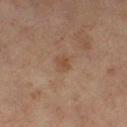Captured during whole-body skin photography for melanoma surveillance; the lesion was not biopsied.
From the left thigh.
This image is a 15 mm lesion crop taken from a total-body photograph.
Approximately 2.5 mm at its widest.
This is a cross-polarized tile.
The patient is a female roughly 60 years of age.